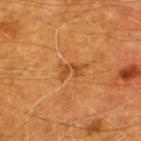workup: catalogued during a skin exam; not biopsied | image source: ~15 mm crop, total-body skin-cancer survey | patient: male, aged around 60 | location: the upper back | lesion diameter: about 3 mm | TBP lesion metrics: a mean CIELAB color near L≈45 a*≈26 b*≈41, roughly 8 lightness units darker than nearby skin, and a lesion-to-skin contrast of about 6.5 (normalized; higher = more distinct); border irregularity of about 5.5 on a 0–10 scale and internal color variation of about 0 on a 0–10 scale; a classifier nevus-likeness of about 0/100 and a lesion-detection confidence of about 100/100.